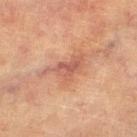{
  "biopsy_status": "not biopsied; imaged during a skin examination",
  "image": {
    "source": "total-body photography crop",
    "field_of_view_mm": 15
  },
  "lesion_size": {
    "long_diameter_mm_approx": 5.5
  },
  "patient": {
    "sex": "female",
    "age_approx": 80
  },
  "automated_metrics": {
    "area_mm2_approx": 8.0,
    "eccentricity": 0.85,
    "shape_asymmetry": 0.5,
    "vs_skin_darker_L": 9.0,
    "vs_skin_contrast_norm": 6.5
  },
  "site": "left lower leg",
  "lighting": "cross-polarized"
}